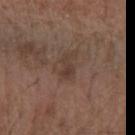follow-up = no biopsy performed (imaged during a skin exam) | lighting = white-light illumination | body site = the left forearm | imaging modality = ~15 mm tile from a whole-body skin photo | patient = male, in their mid- to late 50s.